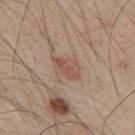| key | value |
|---|---|
| follow-up | catalogued during a skin exam; not biopsied |
| site | the mid back |
| patient | male, aged around 50 |
| imaging modality | ~15 mm tile from a whole-body skin photo |
| image-analysis metrics | a lesion area of about 5 mm²; an average lesion color of about L≈52 a*≈20 b*≈27 (CIELAB), a lesion–skin lightness drop of about 8, and a normalized lesion–skin contrast near 6; a within-lesion color-variation index near 2/10; a nevus-likeness score of about 20/100 and lesion-presence confidence of about 100/100 |
| size | ≈3.5 mm |
| illumination | white-light |